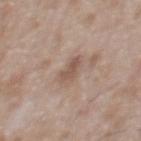Case summary:
- workup: total-body-photography surveillance lesion; no biopsy
- anatomic site: the front of the torso
- lighting: white-light illumination
- diameter: about 2.5 mm
- automated metrics: a lesion area of about 4 mm²; a normalized lesion–skin contrast near 6.5
- subject: male, aged 63 to 67
- imaging modality: 15 mm crop, total-body photography